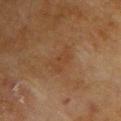Q: Is there a histopathology result?
A: no biopsy performed (imaged during a skin exam)
Q: Automated lesion metrics?
A: a lesion color around L≈36 a*≈18 b*≈29 in CIELAB, a lesion–skin lightness drop of about 5, and a lesion-to-skin contrast of about 4.5 (normalized; higher = more distinct); a color-variation rating of about 1.5/10 and a peripheral color-asymmetry measure near 0.5; an automated nevus-likeness rating near 0 out of 100 and a lesion-detection confidence of about 100/100
Q: Illumination type?
A: cross-polarized
Q: Who is the patient?
A: female, aged 78–82
Q: Lesion location?
A: the back
Q: What kind of image is this?
A: 15 mm crop, total-body photography
Q: How large is the lesion?
A: ~3.5 mm (longest diameter)